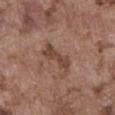– notes: total-body-photography surveillance lesion; no biopsy
– image: total-body-photography crop, ~15 mm field of view
– size: ~4.5 mm (longest diameter)
– illumination: white-light
– subject: male, aged 73 to 77
– automated lesion analysis: an outline eccentricity of about 0.9 (0 = round, 1 = elongated) and a shape-asymmetry score of about 0.25 (0 = symmetric); an average lesion color of about L≈44 a*≈19 b*≈25 (CIELAB), roughly 9 lightness units darker than nearby skin, and a normalized border contrast of about 7.5
– anatomic site: the abdomen